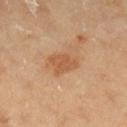Q: Was this lesion biopsied?
A: no biopsy performed (imaged during a skin exam)
Q: What is the lesion's diameter?
A: ≈4 mm
Q: Who is the patient?
A: female, about 60 years old
Q: What did automated image analysis measure?
A: a lesion color around L≈57 a*≈23 b*≈38 in CIELAB and roughly 9 lightness units darker than nearby skin
Q: Lesion location?
A: the right lower leg
Q: How was this image acquired?
A: 15 mm crop, total-body photography
Q: What lighting was used for the tile?
A: cross-polarized illumination